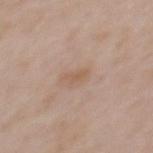A 15 mm close-up tile from a total-body photography series done for melanoma screening.
On the back.
A female patient, roughly 30 years of age.